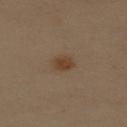Clinical impression: This lesion was catalogued during total-body skin photography and was not selected for biopsy. Background: Automated tile analysis of the lesion measured a lesion color around L≈34 a*≈13 b*≈25 in CIELAB, about 6 CIELAB-L* units darker than the surrounding skin, and a normalized border contrast of about 7.5. The software also gave border irregularity of about 1.5 on a 0–10 scale and peripheral color asymmetry of about 0.5. It also reported a nevus-likeness score of about 95/100. A 15 mm crop from a total-body photograph taken for skin-cancer surveillance. On the back. Measured at roughly 3 mm in maximum diameter. Imaged with cross-polarized lighting. A female patient in their 60s.